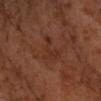Recorded during total-body skin imaging; not selected for excision or biopsy. An algorithmic analysis of the crop reported an average lesion color of about L≈28 a*≈21 b*≈26 (CIELAB), a lesion–skin lightness drop of about 4, and a lesion-to-skin contrast of about 5 (normalized; higher = more distinct). It also reported a classifier nevus-likeness of about 0/100 and lesion-presence confidence of about 95/100. Imaged with cross-polarized lighting. The patient is a male approximately 60 years of age. Measured at roughly 4 mm in maximum diameter. From the right forearm. This image is a 15 mm lesion crop taken from a total-body photograph.